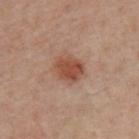Captured during whole-body skin photography for melanoma surveillance; the lesion was not biopsied. A male subject aged approximately 40. The lesion is on the chest. A 15 mm close-up extracted from a 3D total-body photography capture. An algorithmic analysis of the crop reported an area of roughly 8 mm², a shape eccentricity near 0.5, and two-axis asymmetry of about 0.2. The analysis additionally found an average lesion color of about L≈38 a*≈19 b*≈24 (CIELAB), a lesion–skin lightness drop of about 9, and a lesion-to-skin contrast of about 8 (normalized; higher = more distinct). The analysis additionally found a nevus-likeness score of about 85/100 and a lesion-detection confidence of about 100/100. The tile uses cross-polarized illumination.